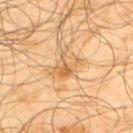follow-up — total-body-photography surveillance lesion; no biopsy | tile lighting — cross-polarized | patient — male, aged approximately 65 | TBP lesion metrics — an area of roughly 7 mm² and a shape eccentricity near 0.7; a lesion color around L≈62 a*≈19 b*≈41 in CIELAB and roughly 9 lightness units darker than nearby skin; a classifier nevus-likeness of about 0/100 and lesion-presence confidence of about 100/100 | site — the upper back | image — total-body-photography crop, ~15 mm field of view.